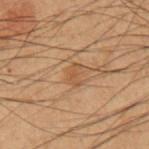notes — total-body-photography surveillance lesion; no biopsy
site — the left forearm
lighting — cross-polarized illumination
subject — male, in their mid- to late 50s
automated lesion analysis — a lesion area of about 3.5 mm² and two-axis asymmetry of about 0.35; a lesion color around L≈42 a*≈17 b*≈30 in CIELAB, about 6 CIELAB-L* units darker than the surrounding skin, and a normalized lesion–skin contrast near 5
image source — ~15 mm tile from a whole-body skin photo